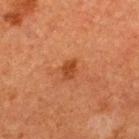Impression:
No biopsy was performed on this lesion — it was imaged during a full skin examination and was not determined to be concerning.
Background:
A 15 mm crop from a total-body photograph taken for skin-cancer surveillance. Imaged with cross-polarized lighting. From the upper back. A male subject aged around 50.